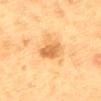Assessment:
The lesion was tiled from a total-body skin photograph and was not biopsied.
Context:
The patient is a male roughly 60 years of age. The tile uses cross-polarized illumination. This image is a 15 mm lesion crop taken from a total-body photograph. On the mid back. Measured at roughly 3.5 mm in maximum diameter. Automated image analysis of the tile measured an outline eccentricity of about 0.85 (0 = round, 1 = elongated). It also reported an average lesion color of about L≈66 a*≈25 b*≈48 (CIELAB), a lesion–skin lightness drop of about 13, and a normalized lesion–skin contrast near 7.5.